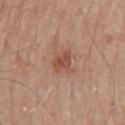<tbp_lesion>
  <biopsy_status>not biopsied; imaged during a skin examination</biopsy_status>
  <site>mid back</site>
  <patient>
    <sex>male</sex>
    <age_approx>75</age_approx>
  </patient>
  <image>
    <source>total-body photography crop</source>
    <field_of_view_mm>15</field_of_view_mm>
  </image>
  <lesion_size>
    <long_diameter_mm_approx>2.5</long_diameter_mm_approx>
  </lesion_size>
</tbp_lesion>